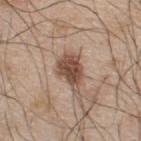Context:
A roughly 15 mm field-of-view crop from a total-body skin photograph. This is a white-light tile. A male subject, in their mid- to late 40s. Automated image analysis of the tile measured a lesion area of about 8.5 mm², an eccentricity of roughly 0.7, and a shape-asymmetry score of about 0.3 (0 = symmetric). It also reported a border-irregularity index near 3/10, a color-variation rating of about 3.5/10, and radial color variation of about 1. The software also gave an automated nevus-likeness rating near 85 out of 100. From the upper back. Longest diameter approximately 4 mm.